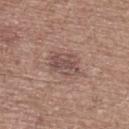<case>
  <biopsy_status>not biopsied; imaged during a skin examination</biopsy_status>
  <lesion_size>
    <long_diameter_mm_approx>3.5</long_diameter_mm_approx>
  </lesion_size>
  <patient>
    <sex>male</sex>
    <age_approx>60</age_approx>
  </patient>
  <site>upper back</site>
  <automated_metrics>
    <area_mm2_approx>9.0</area_mm2_approx>
    <eccentricity>0.65</eccentricity>
    <shape_asymmetry>0.25</shape_asymmetry>
    <border_irregularity_0_10>2.0</border_irregularity_0_10>
    <peripheral_color_asymmetry>1.0</peripheral_color_asymmetry>
    <nevus_likeness_0_100>0</nevus_likeness_0_100>
    <lesion_detection_confidence_0_100>100</lesion_detection_confidence_0_100>
  </automated_metrics>
  <image>
    <source>total-body photography crop</source>
    <field_of_view_mm>15</field_of_view_mm>
  </image>
  <lighting>white-light</lighting>
</case>